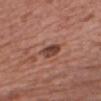biopsy status: imaged on a skin check; not biopsied
automated lesion analysis: an area of roughly 5 mm² and a symmetry-axis asymmetry near 0.25; a lesion color around L≈43 a*≈23 b*≈27 in CIELAB and a lesion-to-skin contrast of about 9.5 (normalized; higher = more distinct); a nevus-likeness score of about 10/100 and a detector confidence of about 100 out of 100 that the crop contains a lesion
lighting: white-light
anatomic site: the chest
patient: male, aged around 60
lesion size: ~3 mm (longest diameter)
acquisition: total-body-photography crop, ~15 mm field of view